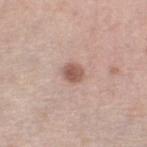– workup — no biopsy performed (imaged during a skin exam)
– acquisition — ~15 mm tile from a whole-body skin photo
– illumination — white-light illumination
– subject — female, approximately 65 years of age
– lesion size — about 2.5 mm
– site — the left thigh